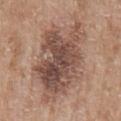Assessment:
Part of a total-body skin-imaging series; this lesion was reviewed on a skin check and was not flagged for biopsy.
Image and clinical context:
Measured at roughly 12.5 mm in maximum diameter. This image is a 15 mm lesion crop taken from a total-body photograph. The lesion-visualizer software estimated a footprint of about 50 mm², an outline eccentricity of about 0.85 (0 = round, 1 = elongated), and two-axis asymmetry of about 0.25. The software also gave a border-irregularity index near 4.5/10, a within-lesion color-variation index near 8/10, and radial color variation of about 2.5. Imaged with white-light lighting. The lesion is located on the left upper arm. A female subject, about 85 years old or older.